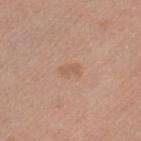The lesion was photographed on a routine skin check and not biopsied; there is no pathology result.
The patient is a female roughly 55 years of age.
On the left thigh.
This image is a 15 mm lesion crop taken from a total-body photograph.